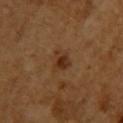Impression:
Recorded during total-body skin imaging; not selected for excision or biopsy.
Context:
The subject is a female aged 53–57. The tile uses cross-polarized illumination. The lesion's longest dimension is about 3 mm. From the upper back. Cropped from a whole-body photographic skin survey; the tile spans about 15 mm.